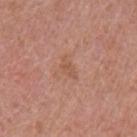workup: no biopsy performed (imaged during a skin exam)
location: the arm
patient: female, in their 40s
image-analysis metrics: a lesion area of about 3 mm² and a symmetry-axis asymmetry near 0.35; a lesion color around L≈54 a*≈22 b*≈31 in CIELAB and a normalized lesion–skin contrast near 5
image source: total-body-photography crop, ~15 mm field of view
illumination: white-light